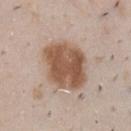follow-up: imaged on a skin check; not biopsied | anatomic site: the chest | lighting: white-light | subject: male, roughly 50 years of age | lesion size: ~6 mm (longest diameter) | acquisition: total-body-photography crop, ~15 mm field of view.